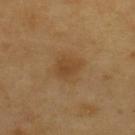biopsy_status: not biopsied; imaged during a skin examination